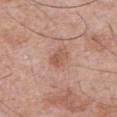Clinical impression:
This lesion was catalogued during total-body skin photography and was not selected for biopsy.
Background:
Automated image analysis of the tile measured an area of roughly 4 mm², an eccentricity of roughly 0.8, and a shape-asymmetry score of about 0.2 (0 = symmetric). And it measured a mean CIELAB color near L≈55 a*≈22 b*≈28, roughly 9 lightness units darker than nearby skin, and a normalized border contrast of about 6. The analysis additionally found border irregularity of about 2 on a 0–10 scale, a color-variation rating of about 3.5/10, and a peripheral color-asymmetry measure near 1. And it measured a lesion-detection confidence of about 100/100. Approximately 2.5 mm at its widest. The lesion is located on the abdomen. A male subject, aged 68 to 72. A roughly 15 mm field-of-view crop from a total-body skin photograph.